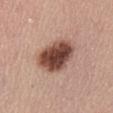Q: Is there a histopathology result?
A: catalogued during a skin exam; not biopsied
Q: Patient demographics?
A: female, in their mid-40s
Q: Automated lesion metrics?
A: a lesion area of about 17 mm²; a nevus-likeness score of about 45/100 and a lesion-detection confidence of about 100/100
Q: How was the tile lit?
A: white-light illumination
Q: What is the imaging modality?
A: ~15 mm tile from a whole-body skin photo
Q: Lesion location?
A: the abdomen
Q: What is the lesion's diameter?
A: about 5 mm